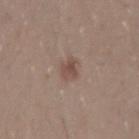Part of a total-body skin-imaging series; this lesion was reviewed on a skin check and was not flagged for biopsy. Located on the right upper arm. An algorithmic analysis of the crop reported a nevus-likeness score of about 55/100 and a lesion-detection confidence of about 100/100. The subject is a male approximately 30 years of age. A 15 mm close-up tile from a total-body photography series done for melanoma screening. Imaged with white-light lighting. The lesion's longest dimension is about 2.5 mm.